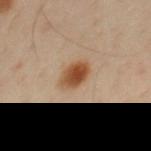Assessment: The lesion was photographed on a routine skin check and not biopsied; there is no pathology result. Image and clinical context: Measured at roughly 3.5 mm in maximum diameter. The lesion is on the chest. A male patient, approximately 40 years of age. A 15 mm crop from a total-body photograph taken for skin-cancer surveillance.